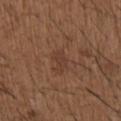The lesion was tiled from a total-body skin photograph and was not biopsied. Cropped from a whole-body photographic skin survey; the tile spans about 15 mm. The total-body-photography lesion software estimated a border-irregularity index near 3.5/10 and a within-lesion color-variation index near 1/10. The analysis additionally found a nevus-likeness score of about 0/100 and a detector confidence of about 100 out of 100 that the crop contains a lesion. Located on the right upper arm. The recorded lesion diameter is about 2.5 mm. A male subject approximately 50 years of age. Imaged with white-light lighting.